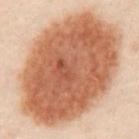Impression:
Part of a total-body skin-imaging series; this lesion was reviewed on a skin check and was not flagged for biopsy.
Context:
The tile uses cross-polarized illumination. From the abdomen. The lesion-visualizer software estimated a shape eccentricity near 0.7. It also reported a border-irregularity index near 1/10, internal color variation of about 5 on a 0–10 scale, and a peripheral color-asymmetry measure near 2. The software also gave a nevus-likeness score of about 100/100 and lesion-presence confidence of about 100/100. Cropped from a whole-body photographic skin survey; the tile spans about 15 mm. Longest diameter approximately 13.5 mm. A male subject aged 48 to 52.